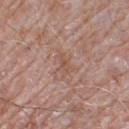  biopsy_status: not biopsied; imaged during a skin examination
  patient:
    sex: male
    age_approx: 75
  lesion_size:
    long_diameter_mm_approx: 3.0
  automated_metrics:
    vs_skin_contrast_norm: 5.0
    border_irregularity_0_10: 4.5
    color_variation_0_10: 2.5
    peripheral_color_asymmetry: 0.5
  site: left lower leg
  image:
    source: total-body photography crop
    field_of_view_mm: 15
  lighting: white-light